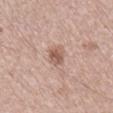follow-up = no biopsy performed (imaged during a skin exam); imaging modality = ~15 mm crop, total-body skin-cancer survey; site = the leg; patient = male, aged approximately 50; lighting = white-light.